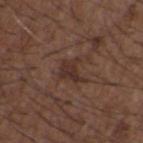Q: Was a biopsy performed?
A: catalogued during a skin exam; not biopsied
Q: Who is the patient?
A: male, aged 48–52
Q: Lesion location?
A: the back
Q: What kind of image is this?
A: total-body-photography crop, ~15 mm field of view